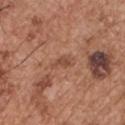The lesion was photographed on a routine skin check and not biopsied; there is no pathology result.
A 15 mm crop from a total-body photograph taken for skin-cancer surveillance.
The lesion is on the chest.
A male patient, aged 53–57.